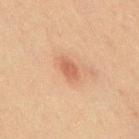Clinical impression:
Part of a total-body skin-imaging series; this lesion was reviewed on a skin check and was not flagged for biopsy.
Background:
Imaged with cross-polarized lighting. Automated image analysis of the tile measured an average lesion color of about L≈49 a*≈22 b*≈29 (CIELAB), a lesion–skin lightness drop of about 8, and a normalized border contrast of about 6.5. The subject is a male approximately 70 years of age. On the chest. About 3 mm across. A roughly 15 mm field-of-view crop from a total-body skin photograph.